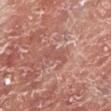Assessment:
Recorded during total-body skin imaging; not selected for excision or biopsy.